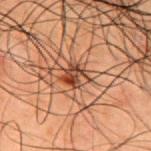notes: imaged on a skin check; not biopsied | TBP lesion metrics: a border-irregularity index near 3/10 and a within-lesion color-variation index near 7.5/10 | lesion diameter: about 2.5 mm | tile lighting: cross-polarized illumination | subject: male, aged approximately 50 | imaging modality: ~15 mm crop, total-body skin-cancer survey | body site: the upper back.